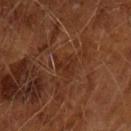This lesion was catalogued during total-body skin photography and was not selected for biopsy.
Measured at roughly 3 mm in maximum diameter.
A lesion tile, about 15 mm wide, cut from a 3D total-body photograph.
This is a cross-polarized tile.
The total-body-photography lesion software estimated an average lesion color of about L≈28 a*≈22 b*≈29 (CIELAB) and a normalized border contrast of about 5.5. It also reported a border-irregularity rating of about 6/10, a color-variation rating of about 1.5/10, and radial color variation of about 0.5.
A male subject aged 63 to 67.